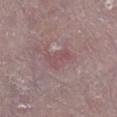TBP lesion metrics=a lesion color around L≈50 a*≈20 b*≈16 in CIELAB, roughly 6 lightness units darker than nearby skin, and a normalized border contrast of about 4.5; a classifier nevus-likeness of about 0/100 and a detector confidence of about 90 out of 100 that the crop contains a lesion | patient=male, roughly 65 years of age | size=about 3.5 mm | acquisition=15 mm crop, total-body photography | site=the right lower leg | lighting=white-light illumination.